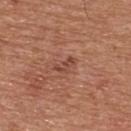biopsy status: imaged on a skin check; not biopsied | imaging modality: ~15 mm tile from a whole-body skin photo | subject: male, approximately 65 years of age | location: the upper back.